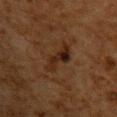The lesion was tiled from a total-body skin photograph and was not biopsied. A close-up tile cropped from a whole-body skin photograph, about 15 mm across. The lesion is on the upper back. The patient is a male aged 58 to 62.